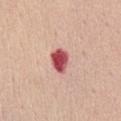Q: Is there a histopathology result?
A: no biopsy performed (imaged during a skin exam)
Q: What is the imaging modality?
A: total-body-photography crop, ~15 mm field of view
Q: Lesion location?
A: the abdomen
Q: What are the patient's age and sex?
A: female, aged 48–52